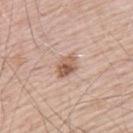illumination = white-light | subject = male, about 70 years old | diameter = about 3 mm | anatomic site = the upper back | imaging modality = 15 mm crop, total-body photography.